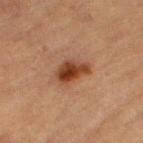Notes:
- notes · imaged on a skin check; not biopsied
- site · the left thigh
- image · ~15 mm crop, total-body skin-cancer survey
- patient · male, aged 83 or over